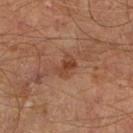The lesion-visualizer software estimated a lesion–skin lightness drop of about 8. A lesion tile, about 15 mm wide, cut from a 3D total-body photograph. The lesion is located on the right lower leg. This is a cross-polarized tile. A male subject, aged approximately 65.